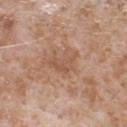Notes:
• workup: total-body-photography surveillance lesion; no biopsy
• patient: male, approximately 65 years of age
• imaging modality: ~15 mm crop, total-body skin-cancer survey
• lesion diameter: ≈3.5 mm
• site: the chest
• tile lighting: white-light